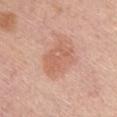The lesion was tiled from a total-body skin photograph and was not biopsied. The lesion is on the chest. This image is a 15 mm lesion crop taken from a total-body photograph. Longest diameter approximately 5 mm. Automated image analysis of the tile measured a lesion area of about 11 mm², an eccentricity of roughly 0.75, and a shape-asymmetry score of about 0.2 (0 = symmetric). It also reported a mean CIELAB color near L≈61 a*≈24 b*≈31, a lesion–skin lightness drop of about 8, and a normalized lesion–skin contrast near 5.5. The software also gave internal color variation of about 2.5 on a 0–10 scale and a peripheral color-asymmetry measure near 1. The software also gave a lesion-detection confidence of about 100/100. A female patient, aged 53 to 57. The tile uses white-light illumination.